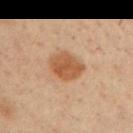Q: Was this lesion biopsied?
A: no biopsy performed (imaged during a skin exam)
Q: How was the tile lit?
A: cross-polarized
Q: Patient demographics?
A: male, aged 33–37
Q: Lesion size?
A: ~4 mm (longest diameter)
Q: What is the imaging modality?
A: total-body-photography crop, ~15 mm field of view
Q: Where on the body is the lesion?
A: the left upper arm